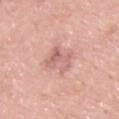biopsy status — total-body-photography surveillance lesion; no biopsy
imaging modality — ~15 mm tile from a whole-body skin photo
lighting — white-light
site — the chest
TBP lesion metrics — a lesion area of about 7.5 mm² and a shape eccentricity near 0.8; a lesion-to-skin contrast of about 6 (normalized; higher = more distinct); border irregularity of about 4.5 on a 0–10 scale and radial color variation of about 1.5
diameter — ~4 mm (longest diameter)
patient — male, roughly 50 years of age